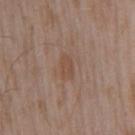No biopsy was performed on this lesion — it was imaged during a full skin examination and was not determined to be concerning. The recorded lesion diameter is about 3 mm. The lesion-visualizer software estimated an eccentricity of roughly 0.85 and two-axis asymmetry of about 0.3. And it measured a border-irregularity index near 3/10 and internal color variation of about 1.5 on a 0–10 scale. The subject is a male aged approximately 55. This image is a 15 mm lesion crop taken from a total-body photograph. The lesion is located on the arm.